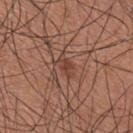Part of a total-body skin-imaging series; this lesion was reviewed on a skin check and was not flagged for biopsy. A male subject, in their mid- to late 30s. This is a white-light tile. An algorithmic analysis of the crop reported a lesion area of about 4 mm², a shape eccentricity near 0.85, and a shape-asymmetry score of about 0.35 (0 = symmetric). And it measured a border-irregularity index near 4/10, a color-variation rating of about 2.5/10, and radial color variation of about 1. And it measured an automated nevus-likeness rating near 10 out of 100 and a lesion-detection confidence of about 95/100. Measured at roughly 3 mm in maximum diameter. The lesion is located on the right upper arm. A 15 mm crop from a total-body photograph taken for skin-cancer surveillance.